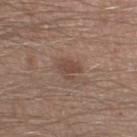Q: Is there a histopathology result?
A: catalogued during a skin exam; not biopsied
Q: What are the patient's age and sex?
A: male, aged around 35
Q: What is the anatomic site?
A: the leg
Q: How was this image acquired?
A: ~15 mm crop, total-body skin-cancer survey
Q: What did automated image analysis measure?
A: an area of roughly 5.5 mm²; a nevus-likeness score of about 15/100 and lesion-presence confidence of about 100/100
Q: What lighting was used for the tile?
A: white-light